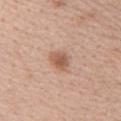workup: no biopsy performed (imaged during a skin exam) | image-analysis metrics: a footprint of about 5 mm², an outline eccentricity of about 0.65 (0 = round, 1 = elongated), and two-axis asymmetry of about 0.25; a border-irregularity rating of about 2.5/10, a color-variation rating of about 3/10, and a peripheral color-asymmetry measure near 1; a nevus-likeness score of about 90/100 and lesion-presence confidence of about 100/100 | location: the back | acquisition: 15 mm crop, total-body photography | subject: female, roughly 40 years of age | diameter: about 3 mm.